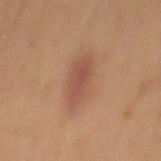Notes:
- workup · catalogued during a skin exam; not biopsied